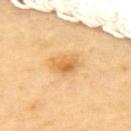The lesion-visualizer software estimated an average lesion color of about L≈69 a*≈21 b*≈49 (CIELAB) and a lesion–skin lightness drop of about 10. The software also gave a border-irregularity index near 2/10 and peripheral color asymmetry of about 2. The software also gave an automated nevus-likeness rating near 75 out of 100 and a lesion-detection confidence of about 100/100.
From the upper back.
A female patient aged 68 to 72.
A 15 mm crop from a total-body photograph taken for skin-cancer surveillance.
Longest diameter approximately 3 mm.
Imaged with cross-polarized lighting.